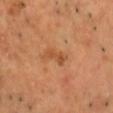follow-up: no biopsy performed (imaged during a skin exam); image source: ~15 mm crop, total-body skin-cancer survey; lesion diameter: ~3 mm (longest diameter); subject: male, aged around 50; illumination: cross-polarized; body site: the chest.